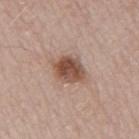Q: Is there a histopathology result?
A: catalogued during a skin exam; not biopsied
Q: What kind of image is this?
A: 15 mm crop, total-body photography
Q: What is the anatomic site?
A: the mid back
Q: What are the patient's age and sex?
A: male, approximately 65 years of age